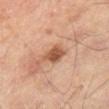Q: Was this lesion biopsied?
A: imaged on a skin check; not biopsied
Q: How large is the lesion?
A: ≈3 mm
Q: What did automated image analysis measure?
A: a border-irregularity rating of about 2.5/10, a within-lesion color-variation index near 3/10, and peripheral color asymmetry of about 1; a classifier nevus-likeness of about 70/100 and a lesion-detection confidence of about 100/100
Q: What is the anatomic site?
A: the right lower leg
Q: What are the patient's age and sex?
A: male, in their mid-60s
Q: What is the imaging modality?
A: 15 mm crop, total-body photography